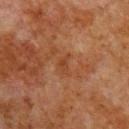<lesion>
  <biopsy_status>not biopsied; imaged during a skin examination</biopsy_status>
  <site>right upper arm</site>
  <image>
    <source>total-body photography crop</source>
    <field_of_view_mm>15</field_of_view_mm>
  </image>
  <patient>
    <sex>male</sex>
    <age_approx>80</age_approx>
  </patient>
</lesion>